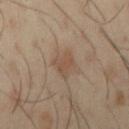The lesion was photographed on a routine skin check and not biopsied; there is no pathology result.
The recorded lesion diameter is about 2.5 mm.
The tile uses cross-polarized illumination.
This image is a 15 mm lesion crop taken from a total-body photograph.
The total-body-photography lesion software estimated an eccentricity of roughly 0.55 and two-axis asymmetry of about 0.4. The software also gave a mean CIELAB color near L≈48 a*≈16 b*≈27, a lesion–skin lightness drop of about 6, and a lesion-to-skin contrast of about 5.5 (normalized; higher = more distinct).
A male subject in their 40s.
From the right upper arm.